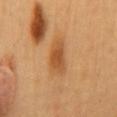Case summary:
* notes — imaged on a skin check; not biopsied
* automated lesion analysis — an area of roughly 8.5 mm², an outline eccentricity of about 0.9 (0 = round, 1 = elongated), and a shape-asymmetry score of about 0.25 (0 = symmetric); about 11 CIELAB-L* units darker than the surrounding skin; a border-irregularity index near 2.5/10 and a color-variation rating of about 4/10
* imaging modality — ~15 mm crop, total-body skin-cancer survey
* patient — female, aged around 60
* anatomic site — the mid back
* tile lighting — cross-polarized illumination
* diameter — ≈4.5 mm